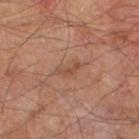Assessment: Recorded during total-body skin imaging; not selected for excision or biopsy. Image and clinical context: An algorithmic analysis of the crop reported a footprint of about 1.5 mm² and a shape-asymmetry score of about 0.65 (0 = symmetric). And it measured a border-irregularity rating of about 7/10, internal color variation of about 0 on a 0–10 scale, and peripheral color asymmetry of about 0. The lesion is on the left lower leg. A male subject, aged 63 to 67. Cropped from a total-body skin-imaging series; the visible field is about 15 mm. Longest diameter approximately 2.5 mm.